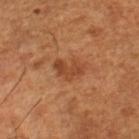| field | value |
|---|---|
| workup | total-body-photography surveillance lesion; no biopsy |
| lighting | cross-polarized |
| subject | male, aged 63 to 67 |
| acquisition | ~15 mm tile from a whole-body skin photo |
| automated metrics | a border-irregularity rating of about 4.5/10 and a peripheral color-asymmetry measure near 1 |
| location | the right lower leg |
| lesion size | ~3.5 mm (longest diameter) |